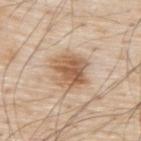Imaged during a routine full-body skin examination; the lesion was not biopsied and no histopathology is available.
A 15 mm close-up tile from a total-body photography series done for melanoma screening.
From the upper back.
Approximately 4.5 mm at its widest.
A male subject in their 80s.